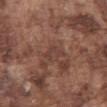A male subject, roughly 75 years of age. From the abdomen. A close-up tile cropped from a whole-body skin photograph, about 15 mm across.